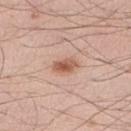workup: imaged on a skin check; not biopsied
image-analysis metrics: border irregularity of about 2.5 on a 0–10 scale; a nevus-likeness score of about 95/100 and lesion-presence confidence of about 100/100
acquisition: ~15 mm tile from a whole-body skin photo
illumination: white-light
site: the right lower leg
subject: male, roughly 25 years of age